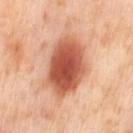Captured during whole-body skin photography for melanoma surveillance; the lesion was not biopsied. The lesion's longest dimension is about 8.5 mm. Imaged with cross-polarized lighting. A female subject, in their mid-50s. Cropped from a whole-body photographic skin survey; the tile spans about 15 mm. The lesion is located on the left thigh.